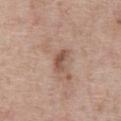Captured during whole-body skin photography for melanoma surveillance; the lesion was not biopsied. The lesion is located on the chest. A roughly 15 mm field-of-view crop from a total-body skin photograph. Longest diameter approximately 3 mm. Automated tile analysis of the lesion measured an average lesion color of about L≈52 a*≈19 b*≈27 (CIELAB) and a lesion–skin lightness drop of about 11. It also reported an automated nevus-likeness rating near 5 out of 100 and lesion-presence confidence of about 100/100. Captured under white-light illumination. A male subject, in their 60s.